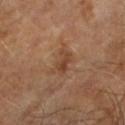Clinical impression: Captured during whole-body skin photography for melanoma surveillance; the lesion was not biopsied. Clinical summary: The recorded lesion diameter is about 3.5 mm. A male subject aged around 65. The tile uses cross-polarized illumination. A region of skin cropped from a whole-body photographic capture, roughly 15 mm wide.